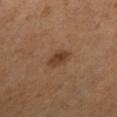image source: ~15 mm crop, total-body skin-cancer survey
lighting: cross-polarized
lesion diameter: ~2.5 mm (longest diameter)
subject: female, approximately 55 years of age
location: the left lower leg